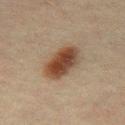notes: catalogued during a skin exam; not biopsied
illumination: cross-polarized illumination
subject: male, aged 48–52
imaging modality: ~15 mm crop, total-body skin-cancer survey
automated metrics: a footprint of about 12 mm², a shape eccentricity near 0.75, and a shape-asymmetry score of about 0.15 (0 = symmetric); a mean CIELAB color near L≈34 a*≈15 b*≈24, a lesion–skin lightness drop of about 12, and a normalized border contrast of about 11; border irregularity of about 1.5 on a 0–10 scale, a within-lesion color-variation index near 5.5/10, and a peripheral color-asymmetry measure near 2; a nevus-likeness score of about 100/100 and a lesion-detection confidence of about 100/100
body site: the abdomen